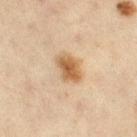Q: Was this lesion biopsied?
A: no biopsy performed (imaged during a skin exam)
Q: What is the imaging modality?
A: ~15 mm tile from a whole-body skin photo
Q: Where on the body is the lesion?
A: the left thigh
Q: Who is the patient?
A: female, aged 43–47
Q: What lighting was used for the tile?
A: cross-polarized